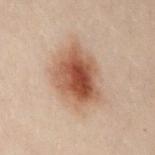biopsy status: no biopsy performed (imaged during a skin exam)
lesion diameter: about 7 mm
subject: female, roughly 30 years of age
location: the chest
image: 15 mm crop, total-body photography
tile lighting: cross-polarized illumination